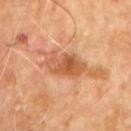No biopsy was performed on this lesion — it was imaged during a full skin examination and was not determined to be concerning.
The lesion is located on the upper back.
The recorded lesion diameter is about 5 mm.
The patient is a male aged around 60.
Cropped from a whole-body photographic skin survey; the tile spans about 15 mm.